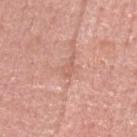Clinical impression:
This lesion was catalogued during total-body skin photography and was not selected for biopsy.